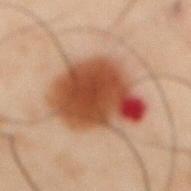No biopsy was performed on this lesion — it was imaged during a full skin examination and was not determined to be concerning. Located on the front of the torso. Imaged with cross-polarized lighting. A 15 mm close-up tile from a total-body photography series done for melanoma screening. A male patient, approximately 50 years of age.